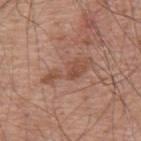follow-up: no biopsy performed (imaged during a skin exam) | acquisition: total-body-photography crop, ~15 mm field of view | subject: male, approximately 55 years of age | size: ~6 mm (longest diameter) | body site: the upper back | illumination: white-light illumination.